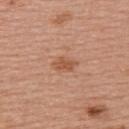{"biopsy_status": "not biopsied; imaged during a skin examination", "image": {"source": "total-body photography crop", "field_of_view_mm": 15}, "site": "upper back", "lighting": "white-light", "patient": {"sex": "female", "age_approx": 55}}